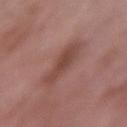body site: the arm
patient: female, approximately 70 years of age
lesion size: ≈5 mm
acquisition: ~15 mm crop, total-body skin-cancer survey
illumination: white-light